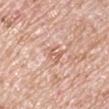Q: Was this lesion biopsied?
A: catalogued during a skin exam; not biopsied
Q: Patient demographics?
A: male, about 70 years old
Q: What is the imaging modality?
A: 15 mm crop, total-body photography
Q: What is the anatomic site?
A: the arm